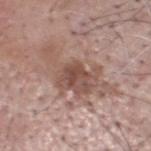The lesion was photographed on a routine skin check and not biopsied; there is no pathology result.
Cropped from a total-body skin-imaging series; the visible field is about 15 mm.
From the head or neck.
A male subject aged 68 to 72.